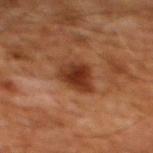This lesion was catalogued during total-body skin photography and was not selected for biopsy. Automated tile analysis of the lesion measured a mean CIELAB color near L≈32 a*≈23 b*≈30, a lesion–skin lightness drop of about 12, and a normalized lesion–skin contrast near 10.5. The analysis additionally found an automated nevus-likeness rating near 95 out of 100 and lesion-presence confidence of about 100/100. The lesion's longest dimension is about 3.5 mm. The patient is a male aged around 60. The lesion is located on the chest. A 15 mm crop from a total-body photograph taken for skin-cancer surveillance.